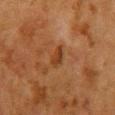Q: Was this lesion biopsied?
A: no biopsy performed (imaged during a skin exam)
Q: Where on the body is the lesion?
A: the front of the torso
Q: What kind of image is this?
A: 15 mm crop, total-body photography
Q: Patient demographics?
A: female, roughly 50 years of age